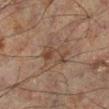| key | value |
|---|---|
| biopsy status | catalogued during a skin exam; not biopsied |
| image | 15 mm crop, total-body photography |
| patient | male, in their 60s |
| TBP lesion metrics | a mean CIELAB color near L≈32 a*≈13 b*≈21; a border-irregularity rating of about 5/10, a color-variation rating of about 3/10, and radial color variation of about 1; a classifier nevus-likeness of about 0/100 and a detector confidence of about 100 out of 100 that the crop contains a lesion |
| location | the left leg |